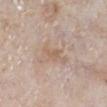Part of a total-body skin-imaging series; this lesion was reviewed on a skin check and was not flagged for biopsy. Automated image analysis of the tile measured an automated nevus-likeness rating near 0 out of 100. This is a white-light tile. A male subject, roughly 80 years of age. A region of skin cropped from a whole-body photographic capture, roughly 15 mm wide. From the left lower leg. The lesion's longest dimension is about 3 mm.